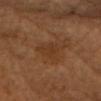Assessment: The lesion was tiled from a total-body skin photograph and was not biopsied. Acquisition and patient details: A female subject, in their 70s. Longest diameter approximately 3.5 mm. From the head or neck. The tile uses cross-polarized illumination. Cropped from a whole-body photographic skin survey; the tile spans about 15 mm.